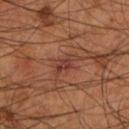Q: Was a biopsy performed?
A: no biopsy performed (imaged during a skin exam)
Q: Lesion location?
A: the left lower leg
Q: What is the imaging modality?
A: ~15 mm crop, total-body skin-cancer survey
Q: Who is the patient?
A: male, aged 53 to 57